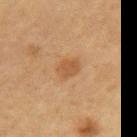| feature | finding |
|---|---|
| lighting | cross-polarized illumination |
| lesion size | about 2.5 mm |
| subject | male, aged 63 to 67 |
| anatomic site | the right upper arm |
| acquisition | 15 mm crop, total-body photography |
| TBP lesion metrics | an area of roughly 4.5 mm², a shape eccentricity near 0.5, and a symmetry-axis asymmetry near 0.2; an average lesion color of about L≈42 a*≈18 b*≈31 (CIELAB), roughly 7 lightness units darker than nearby skin, and a lesion-to-skin contrast of about 6 (normalized; higher = more distinct) |